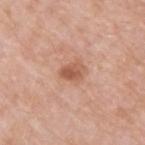This lesion was catalogued during total-body skin photography and was not selected for biopsy.
This is a white-light tile.
A male patient, about 55 years old.
This image is a 15 mm lesion crop taken from a total-body photograph.
Approximately 3 mm at its widest.
On the back.
The total-body-photography lesion software estimated an average lesion color of about L≈56 a*≈24 b*≈32 (CIELAB), roughly 11 lightness units darker than nearby skin, and a lesion-to-skin contrast of about 7.5 (normalized; higher = more distinct). The analysis additionally found border irregularity of about 1.5 on a 0–10 scale, internal color variation of about 4 on a 0–10 scale, and a peripheral color-asymmetry measure near 1.5.